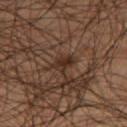The lesion was photographed on a routine skin check and not biopsied; there is no pathology result. The lesion is located on the leg. A close-up tile cropped from a whole-body skin photograph, about 15 mm across. A male patient aged approximately 45. Automated tile analysis of the lesion measured a footprint of about 4.5 mm² and a shape eccentricity near 0.75. It also reported a mean CIELAB color near L≈28 a*≈16 b*≈23, roughly 8 lightness units darker than nearby skin, and a lesion-to-skin contrast of about 8 (normalized; higher = more distinct). And it measured internal color variation of about 3 on a 0–10 scale and radial color variation of about 1.